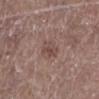{
  "lesion_size": {
    "long_diameter_mm_approx": 3.0
  },
  "site": "leg",
  "image": {
    "source": "total-body photography crop",
    "field_of_view_mm": 15
  },
  "patient": {
    "sex": "male",
    "age_approx": 65
  }
}